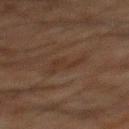Acquisition and patient details:
An algorithmic analysis of the crop reported a lesion area of about 5 mm², an eccentricity of roughly 0.85, and a symmetry-axis asymmetry near 0.55. The software also gave a mean CIELAB color near L≈26 a*≈13 b*≈21 and a lesion–skin lightness drop of about 4. The analysis additionally found a border-irregularity index near 6.5/10 and peripheral color asymmetry of about 0.5. The software also gave a detector confidence of about 100 out of 100 that the crop contains a lesion. The subject is a male aged around 65. Measured at roughly 3.5 mm in maximum diameter. Cropped from a total-body skin-imaging series; the visible field is about 15 mm. The lesion is located on the back. Captured under cross-polarized illumination.